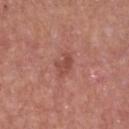Clinical impression:
Captured during whole-body skin photography for melanoma surveillance; the lesion was not biopsied.
Acquisition and patient details:
A male subject roughly 65 years of age. Measured at roughly 2.5 mm in maximum diameter. The lesion is on the chest. Imaged with white-light lighting. Cropped from a total-body skin-imaging series; the visible field is about 15 mm.